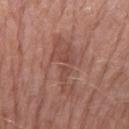notes = no biopsy performed (imaged during a skin exam); TBP lesion metrics = an automated nevus-likeness rating near 0 out of 100 and a detector confidence of about 100 out of 100 that the crop contains a lesion; illumination = white-light illumination; acquisition = total-body-photography crop, ~15 mm field of view; body site = the left forearm; diameter = ~6.5 mm (longest diameter); subject = female, aged approximately 60.